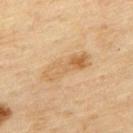<case>
  <biopsy_status>not biopsied; imaged during a skin examination</biopsy_status>
  <patient>
    <sex>male</sex>
    <age_approx>45</age_approx>
  </patient>
  <image>
    <source>total-body photography crop</source>
    <field_of_view_mm>15</field_of_view_mm>
  </image>
  <site>upper back</site>
  <lighting>cross-polarized</lighting>
</case>